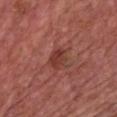No biopsy was performed on this lesion — it was imaged during a full skin examination and was not determined to be concerning.
The lesion is on the chest.
A male subject, aged 73 to 77.
This is a white-light tile.
A region of skin cropped from a whole-body photographic capture, roughly 15 mm wide.
Longest diameter approximately 2.5 mm.
Automated tile analysis of the lesion measured a lesion area of about 4.5 mm² and two-axis asymmetry of about 0.3. The software also gave a lesion color around L≈39 a*≈28 b*≈27 in CIELAB, roughly 8 lightness units darker than nearby skin, and a lesion-to-skin contrast of about 7 (normalized; higher = more distinct). It also reported a within-lesion color-variation index near 3/10 and radial color variation of about 1. The software also gave an automated nevus-likeness rating near 30 out of 100 and lesion-presence confidence of about 100/100.